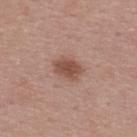This lesion was catalogued during total-body skin photography and was not selected for biopsy.
A roughly 15 mm field-of-view crop from a total-body skin photograph.
The lesion is located on the upper back.
A male subject, approximately 40 years of age.